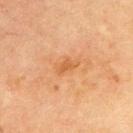Clinical impression: Captured during whole-body skin photography for melanoma surveillance; the lesion was not biopsied.